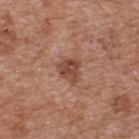notes = no biopsy performed (imaged during a skin exam)
lesion size = ~3 mm (longest diameter)
automated lesion analysis = a lesion area of about 6 mm², a shape eccentricity near 0.6, and a symmetry-axis asymmetry near 0.35
lighting = white-light
anatomic site = the upper back
patient = male, in their mid-60s
image = total-body-photography crop, ~15 mm field of view